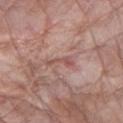The lesion is located on the right forearm.
A close-up tile cropped from a whole-body skin photograph, about 15 mm across.
The patient is a female approximately 65 years of age.